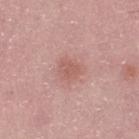The lesion was tiled from a total-body skin photograph and was not biopsied.